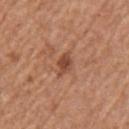biopsy_status: not biopsied; imaged during a skin examination
patient:
  sex: male
  age_approx: 65
lesion_size:
  long_diameter_mm_approx: 2.5
image:
  source: total-body photography crop
  field_of_view_mm: 15
site: arm
lighting: white-light